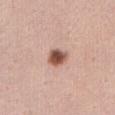biopsy_status: not biopsied; imaged during a skin examination
image:
  source: total-body photography crop
  field_of_view_mm: 15
lesion_size:
  long_diameter_mm_approx: 2.5
patient:
  sex: female
  age_approx: 55
site: front of the torso
lighting: white-light